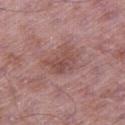  biopsy_status: not biopsied; imaged during a skin examination
  lesion_size:
    long_diameter_mm_approx: 3.5
  automated_metrics:
    area_mm2_approx: 6.5
    eccentricity: 0.75
  image:
    source: total-body photography crop
    field_of_view_mm: 15
  patient:
    sex: male
    age_approx: 50
  lighting: white-light
  site: right thigh